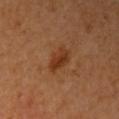Recorded during total-body skin imaging; not selected for excision or biopsy. The tile uses cross-polarized illumination. From the right upper arm. An algorithmic analysis of the crop reported border irregularity of about 3 on a 0–10 scale and a peripheral color-asymmetry measure near 1. The software also gave a classifier nevus-likeness of about 80/100 and lesion-presence confidence of about 100/100. A female subject roughly 40 years of age. A 15 mm close-up tile from a total-body photography series done for melanoma screening.